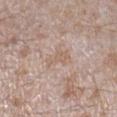Case summary:
• follow-up — imaged on a skin check; not biopsied
• automated lesion analysis — an average lesion color of about L≈60 a*≈16 b*≈27 (CIELAB) and a lesion–skin lightness drop of about 6; a border-irregularity index near 6/10 and radial color variation of about 0
• subject — male, approximately 45 years of age
• imaging modality — ~15 mm tile from a whole-body skin photo
• location — the leg
• lesion size — ≈3 mm